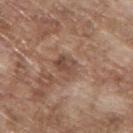Part of a total-body skin-imaging series; this lesion was reviewed on a skin check and was not flagged for biopsy.